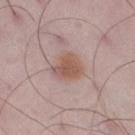The lesion was tiled from a total-body skin photograph and was not biopsied.
The lesion is located on the leg.
The tile uses white-light illumination.
A lesion tile, about 15 mm wide, cut from a 3D total-body photograph.
A male patient, in their 50s.